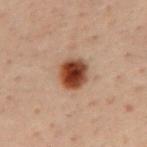Impression: Imaged during a routine full-body skin examination; the lesion was not biopsied and no histopathology is available. Background: The patient is a male aged approximately 50. A 15 mm crop from a total-body photograph taken for skin-cancer surveillance. On the mid back.